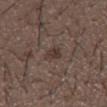Recorded during total-body skin imaging; not selected for excision or biopsy. The recorded lesion diameter is about 3 mm. The tile uses white-light illumination. A male subject in their 50s. The lesion-visualizer software estimated a lesion area of about 3.5 mm², a shape eccentricity near 0.8, and a shape-asymmetry score of about 0.4 (0 = symmetric). It also reported a mean CIELAB color near L≈35 a*≈14 b*≈19, about 7 CIELAB-L* units darker than the surrounding skin, and a normalized border contrast of about 6.5. Cropped from a whole-body photographic skin survey; the tile spans about 15 mm. On the mid back.